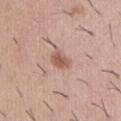Q: Is there a histopathology result?
A: no biopsy performed (imaged during a skin exam)
Q: What did automated image analysis measure?
A: a footprint of about 4.5 mm², a shape eccentricity near 0.7, and a symmetry-axis asymmetry near 0.2; an average lesion color of about L≈57 a*≈21 b*≈28 (CIELAB), roughly 11 lightness units darker than nearby skin, and a normalized border contrast of about 7.5; a border-irregularity index near 2/10, internal color variation of about 2 on a 0–10 scale, and a peripheral color-asymmetry measure near 0.5
Q: What is the imaging modality?
A: ~15 mm tile from a whole-body skin photo
Q: Patient demographics?
A: male, aged 28 to 32
Q: What is the lesion's diameter?
A: about 2.5 mm
Q: Illumination type?
A: white-light
Q: Lesion location?
A: the abdomen